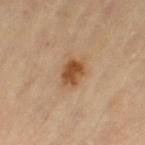Clinical impression: Recorded during total-body skin imaging; not selected for excision or biopsy. Acquisition and patient details: A female patient, aged 68–72. Automated image analysis of the tile measured a classifier nevus-likeness of about 95/100 and a detector confidence of about 100 out of 100 that the crop contains a lesion. This is a cross-polarized tile. Approximately 3.5 mm at its widest. On the left thigh. A 15 mm crop from a total-body photograph taken for skin-cancer surveillance.